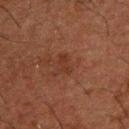Imaged during a routine full-body skin examination; the lesion was not biopsied and no histopathology is available. Located on the upper back. A 15 mm close-up extracted from a 3D total-body photography capture. A male subject aged 48 to 52. The tile uses cross-polarized illumination. Longest diameter approximately 2.5 mm.